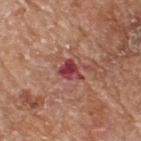Q: Was this lesion biopsied?
A: total-body-photography surveillance lesion; no biopsy
Q: How was this image acquired?
A: 15 mm crop, total-body photography
Q: What is the anatomic site?
A: the upper back
Q: Patient demographics?
A: male, about 65 years old
Q: Lesion size?
A: ≈2.5 mm